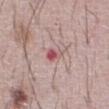{
  "biopsy_status": "not biopsied; imaged during a skin examination",
  "automated_metrics": {
    "area_mm2_approx": 3.5,
    "eccentricity": 0.75,
    "shape_asymmetry": 0.35,
    "cielab_L": 55,
    "cielab_a": 27,
    "cielab_b": 19,
    "vs_skin_darker_L": 11.0,
    "nevus_likeness_0_100": 0,
    "lesion_detection_confidence_0_100": 100
  },
  "patient": {
    "sex": "male",
    "age_approx": 65
  },
  "image": {
    "source": "total-body photography crop",
    "field_of_view_mm": 15
  },
  "lighting": "white-light",
  "lesion_size": {
    "long_diameter_mm_approx": 2.5
  },
  "site": "abdomen"
}